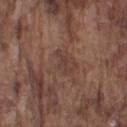Impression:
Imaged during a routine full-body skin examination; the lesion was not biopsied and no histopathology is available.
Acquisition and patient details:
A 15 mm close-up tile from a total-body photography series done for melanoma screening. Located on the left upper arm. Imaged with white-light lighting. A male subject, aged around 75. Automated image analysis of the tile measured an area of roughly 3.5 mm² and an eccentricity of roughly 0.9. It also reported a mean CIELAB color near L≈39 a*≈18 b*≈22 and a lesion–skin lightness drop of about 6. And it measured border irregularity of about 6.5 on a 0–10 scale. And it measured a classifier nevus-likeness of about 0/100 and a detector confidence of about 80 out of 100 that the crop contains a lesion. The recorded lesion diameter is about 3 mm.